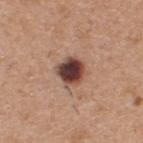notes: catalogued during a skin exam; not biopsied
patient: male, aged approximately 60
acquisition: total-body-photography crop, ~15 mm field of view
size: ~3.5 mm (longest diameter)
site: the upper back